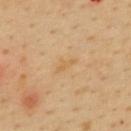biopsy_status: not biopsied; imaged during a skin examination
site: back
patient:
  sex: female
  age_approx: 50
lesion_size:
  long_diameter_mm_approx: 2.5
image:
  source: total-body photography crop
  field_of_view_mm: 15